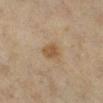{"biopsy_status": "not biopsied; imaged during a skin examination", "patient": {"sex": "female", "age_approx": 40}, "image": {"source": "total-body photography crop", "field_of_view_mm": 15}, "site": "right lower leg", "automated_metrics": {"vs_skin_darker_L": 7.0, "vs_skin_contrast_norm": 7.0, "border_irregularity_0_10": 2.0, "color_variation_0_10": 1.5, "peripheral_color_asymmetry": 0.5}}